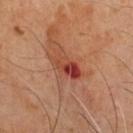Part of a total-body skin-imaging series; this lesion was reviewed on a skin check and was not flagged for biopsy.
Measured at roughly 4 mm in maximum diameter.
Captured under cross-polarized illumination.
A close-up tile cropped from a whole-body skin photograph, about 15 mm across.
A male patient about 65 years old.
From the front of the torso.